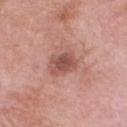No biopsy was performed on this lesion — it was imaged during a full skin examination and was not determined to be concerning.
The lesion is located on the chest.
A male patient, roughly 70 years of age.
An algorithmic analysis of the crop reported a lesion color around L≈52 a*≈24 b*≈25 in CIELAB, a lesion–skin lightness drop of about 11, and a lesion-to-skin contrast of about 7.5 (normalized; higher = more distinct). The analysis additionally found a border-irregularity index near 1.5/10, a color-variation rating of about 3.5/10, and radial color variation of about 1.
Measured at roughly 3.5 mm in maximum diameter.
A 15 mm crop from a total-body photograph taken for skin-cancer surveillance.